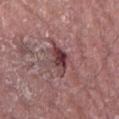Part of a total-body skin-imaging series; this lesion was reviewed on a skin check and was not flagged for biopsy.
The lesion is on the front of the torso.
Captured under white-light illumination.
Longest diameter approximately 4 mm.
A male patient, aged approximately 80.
Cropped from a total-body skin-imaging series; the visible field is about 15 mm.